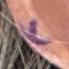On the head or neck.
This image is a 15 mm lesion crop taken from a total-body photograph.
The recorded lesion diameter is about 5.5 mm.
A female patient roughly 55 years of age.
Imaged with white-light lighting.
Automated tile analysis of the lesion measured a border-irregularity index near 8.5/10, internal color variation of about 4.5 on a 0–10 scale, and radial color variation of about 1.5.
Histopathologically confirmed as a dysplastic (Clark) nevus.